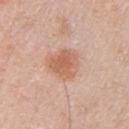The lesion-visualizer software estimated a footprint of about 9.5 mm² and an eccentricity of roughly 0.35. The analysis additionally found border irregularity of about 3 on a 0–10 scale, a color-variation rating of about 3/10, and a peripheral color-asymmetry measure near 1. The analysis additionally found a nevus-likeness score of about 85/100.
The tile uses white-light illumination.
A male patient, aged 78 to 82.
On the arm.
A close-up tile cropped from a whole-body skin photograph, about 15 mm across.